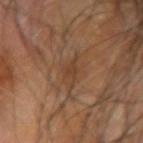Imaged during a routine full-body skin examination; the lesion was not biopsied and no histopathology is available. About 4 mm across. Imaged with cross-polarized lighting. The subject is a male aged approximately 65. A 15 mm close-up tile from a total-body photography series done for melanoma screening. The lesion is located on the left forearm. Automated tile analysis of the lesion measured a lesion area of about 5.5 mm², an outline eccentricity of about 0.8 (0 = round, 1 = elongated), and a shape-asymmetry score of about 0.5 (0 = symmetric). And it measured a mean CIELAB color near L≈42 a*≈18 b*≈30, a lesion–skin lightness drop of about 7, and a lesion-to-skin contrast of about 5.5 (normalized; higher = more distinct). The analysis additionally found a border-irregularity index near 5.5/10, internal color variation of about 2 on a 0–10 scale, and a peripheral color-asymmetry measure near 0.5.